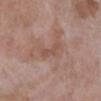Q: Was a biopsy performed?
A: catalogued during a skin exam; not biopsied
Q: What is the lesion's diameter?
A: about 3.5 mm
Q: How was this image acquired?
A: ~15 mm crop, total-body skin-cancer survey
Q: Lesion location?
A: the right lower leg
Q: Who is the patient?
A: female, aged 68 to 72